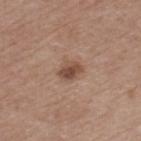biopsy status: catalogued during a skin exam; not biopsied | diameter: about 2.5 mm | automated metrics: roughly 11 lightness units darker than nearby skin and a normalized border contrast of about 8.5 | site: the left thigh | patient: male, approximately 75 years of age | tile lighting: white-light | imaging modality: total-body-photography crop, ~15 mm field of view.